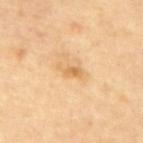Context: The subject is a male about 85 years old or older. Located on the mid back. A 15 mm crop from a total-body photograph taken for skin-cancer surveillance.